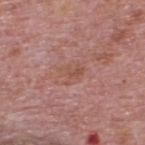Assessment: Captured during whole-body skin photography for melanoma surveillance; the lesion was not biopsied. Clinical summary: Imaged with white-light lighting. The lesion-visualizer software estimated a lesion area of about 5 mm², an eccentricity of roughly 0.6, and a shape-asymmetry score of about 0.35 (0 = symmetric). The analysis additionally found a border-irregularity rating of about 3.5/10. The software also gave an automated nevus-likeness rating near 0 out of 100 and lesion-presence confidence of about 100/100. A male patient in their mid-70s. A 15 mm crop from a total-body photograph taken for skin-cancer surveillance. The lesion is located on the upper back.